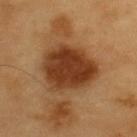biopsy_status: not biopsied; imaged during a skin examination
lighting: cross-polarized
site: back
automated_metrics:
  area_mm2_approx: 28.0
  eccentricity: 0.55
  shape_asymmetry: 0.2
  border_irregularity_0_10: 2.0
  color_variation_0_10: 5.0
  peripheral_color_asymmetry: 1.5
lesion_size:
  long_diameter_mm_approx: 7.0
image:
  source: total-body photography crop
  field_of_view_mm: 15
patient:
  sex: male
  age_approx: 60